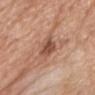| feature | finding |
|---|---|
| workup | imaged on a skin check; not biopsied |